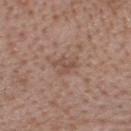{"biopsy_status": "not biopsied; imaged during a skin examination", "lighting": "white-light", "image": {"source": "total-body photography crop", "field_of_view_mm": 15}, "lesion_size": {"long_diameter_mm_approx": 3.0}, "automated_metrics": {"eccentricity": 0.65, "shape_asymmetry": 0.5, "border_irregularity_0_10": 5.5, "peripheral_color_asymmetry": 0.0, "nevus_likeness_0_100": 0, "lesion_detection_confidence_0_100": 100}, "site": "head or neck", "patient": {"sex": "male", "age_approx": 40}}